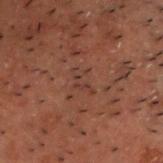Clinical impression:
This lesion was catalogued during total-body skin photography and was not selected for biopsy.
Acquisition and patient details:
A 15 mm crop from a total-body photograph taken for skin-cancer surveillance. The total-body-photography lesion software estimated a lesion area of about 3 mm² and a shape eccentricity near 0.9. It also reported a border-irregularity index near 7/10 and a within-lesion color-variation index near 0/10. And it measured an automated nevus-likeness rating near 0 out of 100 and a lesion-detection confidence of about 70/100. About 3 mm across. On the front of the torso. A male subject about 50 years old. This is a cross-polarized tile.